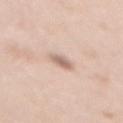biopsy_status: not biopsied; imaged during a skin examination
site: mid back
patient:
  sex: female
  age_approx: 50
lighting: white-light
lesion_size:
  long_diameter_mm_approx: 3.0
image:
  source: total-body photography crop
  field_of_view_mm: 15